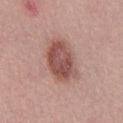Impression:
The lesion was tiled from a total-body skin photograph and was not biopsied.
Background:
A male subject, about 40 years old. Located on the right thigh. This image is a 15 mm lesion crop taken from a total-body photograph.